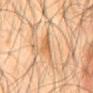Clinical impression: The lesion was tiled from a total-body skin photograph and was not biopsied. Context: The subject is a male aged 63 to 67. Cropped from a whole-body photographic skin survey; the tile spans about 15 mm. This is a cross-polarized tile. Automated image analysis of the tile measured an area of roughly 3 mm², an outline eccentricity of about 0.75 (0 = round, 1 = elongated), and a shape-asymmetry score of about 0.5 (0 = symmetric). The analysis additionally found a classifier nevus-likeness of about 5/100 and a detector confidence of about 95 out of 100 that the crop contains a lesion. The recorded lesion diameter is about 2.5 mm. Located on the mid back.